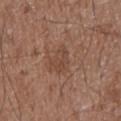{
  "biopsy_status": "not biopsied; imaged during a skin examination",
  "site": "abdomen",
  "image": {
    "source": "total-body photography crop",
    "field_of_view_mm": 15
  },
  "lesion_size": {
    "long_diameter_mm_approx": 3.5
  },
  "automated_metrics": {
    "area_mm2_approx": 6.0,
    "eccentricity": 0.7,
    "shape_asymmetry": 0.35,
    "border_irregularity_0_10": 3.5,
    "color_variation_0_10": 2.5,
    "peripheral_color_asymmetry": 1.0
  },
  "lighting": "white-light",
  "patient": {
    "sex": "male",
    "age_approx": 75
  }
}